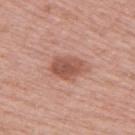biopsy status: catalogued during a skin exam; not biopsied
location: the right upper arm
automated metrics: a border-irregularity rating of about 2/10, a color-variation rating of about 3.5/10, and a peripheral color-asymmetry measure near 1; a classifier nevus-likeness of about 65/100 and a detector confidence of about 100 out of 100 that the crop contains a lesion
imaging modality: ~15 mm tile from a whole-body skin photo
size: about 4 mm
subject: female, in their 60s